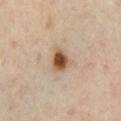Captured during whole-body skin photography for melanoma surveillance; the lesion was not biopsied. On the chest. The tile uses cross-polarized illumination. A region of skin cropped from a whole-body photographic capture, roughly 15 mm wide. Approximately 3 mm at its widest. A male subject, about 65 years old.